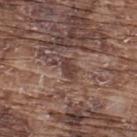Imaged during a routine full-body skin examination; the lesion was not biopsied and no histopathology is available. Cropped from a whole-body photographic skin survey; the tile spans about 15 mm. Automated tile analysis of the lesion measured a mean CIELAB color near L≈39 a*≈18 b*≈22 and a normalized lesion–skin contrast near 8.5. The analysis additionally found a border-irregularity index near 2/10, a color-variation rating of about 1/10, and peripheral color asymmetry of about 0.5. The recorded lesion diameter is about 2.5 mm. On the upper back. The subject is a male in their mid- to late 70s.